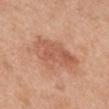Impression: This lesion was catalogued during total-body skin photography and was not selected for biopsy. Image and clinical context: The total-body-photography lesion software estimated a lesion area of about 17 mm² and a shape eccentricity near 0.85. It also reported a mean CIELAB color near L≈58 a*≈25 b*≈32 and a normalized lesion–skin contrast near 6.5. And it measured a detector confidence of about 100 out of 100 that the crop contains a lesion. Approximately 7 mm at its widest. A male subject in their 30s. On the mid back. A close-up tile cropped from a whole-body skin photograph, about 15 mm across.